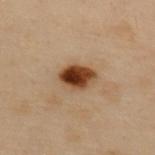Assessment: No biopsy was performed on this lesion — it was imaged during a full skin examination and was not determined to be concerning. Background: A female subject aged 58 to 62. Located on the upper back. The tile uses cross-polarized illumination. A 15 mm crop from a total-body photograph taken for skin-cancer surveillance.